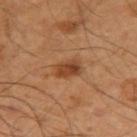Clinical impression:
Imaged during a routine full-body skin examination; the lesion was not biopsied and no histopathology is available.
Background:
Longest diameter approximately 3.5 mm. A 15 mm close-up tile from a total-body photography series done for melanoma screening. The subject is a male about 50 years old. The lesion is located on the left arm. Captured under cross-polarized illumination.